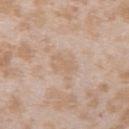The lesion was photographed on a routine skin check and not biopsied; there is no pathology result.
The patient is a female about 25 years old.
Approximately 2.5 mm at its widest.
The lesion is on the upper back.
Imaged with white-light lighting.
The total-body-photography lesion software estimated an area of roughly 3.5 mm², an outline eccentricity of about 0.75 (0 = round, 1 = elongated), and a shape-asymmetry score of about 0.35 (0 = symmetric). The software also gave peripheral color asymmetry of about 0.5.
A lesion tile, about 15 mm wide, cut from a 3D total-body photograph.